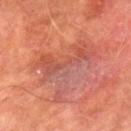Assessment: Captured during whole-body skin photography for melanoma surveillance; the lesion was not biopsied.